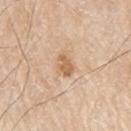Captured during whole-body skin photography for melanoma surveillance; the lesion was not biopsied. Captured under white-light illumination. The lesion is located on the right upper arm. A roughly 15 mm field-of-view crop from a total-body skin photograph. Automated image analysis of the tile measured an area of roughly 4 mm², an outline eccentricity of about 0.8 (0 = round, 1 = elongated), and a shape-asymmetry score of about 0.2 (0 = symmetric). The analysis additionally found roughly 10 lightness units darker than nearby skin and a normalized lesion–skin contrast near 7.5. A male patient, approximately 80 years of age.